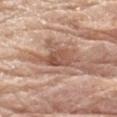Recorded during total-body skin imaging; not selected for excision or biopsy.
Captured under white-light illumination.
The patient is a male in their 80s.
About 5 mm across.
From the arm.
A 15 mm close-up extracted from a 3D total-body photography capture.